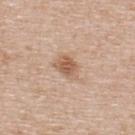biopsy status: catalogued during a skin exam; not biopsied
imaging modality: ~15 mm tile from a whole-body skin photo
body site: the back
lesion size: ≈3 mm
subject: female, aged 38 to 42
illumination: white-light
TBP lesion metrics: a classifier nevus-likeness of about 90/100 and a detector confidence of about 100 out of 100 that the crop contains a lesion